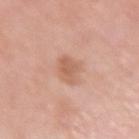follow-up=catalogued during a skin exam; not biopsied
image source=~15 mm tile from a whole-body skin photo
location=the right upper arm
lighting=white-light
size=~3.5 mm (longest diameter)
patient=female, aged 68–72